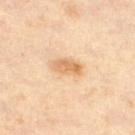Q: Was this lesion biopsied?
A: total-body-photography surveillance lesion; no biopsy